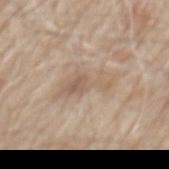{
  "automated_metrics": {
    "area_mm2_approx": 10.0,
    "eccentricity": 0.9,
    "vs_skin_darker_L": 8.0,
    "vs_skin_contrast_norm": 5.5,
    "border_irregularity_0_10": 6.5,
    "peripheral_color_asymmetry": 1.0,
    "nevus_likeness_0_100": 0
  },
  "image": {
    "source": "total-body photography crop",
    "field_of_view_mm": 15
  },
  "site": "mid back",
  "patient": {
    "sex": "male",
    "age_approx": 80
  }
}